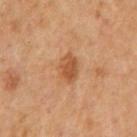| key | value |
|---|---|
| workup | total-body-photography surveillance lesion; no biopsy |
| image-analysis metrics | an average lesion color of about L≈50 a*≈23 b*≈36 (CIELAB), a lesion–skin lightness drop of about 10, and a normalized lesion–skin contrast near 7; a border-irregularity index near 2.5/10 and a peripheral color-asymmetry measure near 1; a detector confidence of about 100 out of 100 that the crop contains a lesion |
| lesion diameter | ≈3.5 mm |
| site | the mid back |
| illumination | cross-polarized |
| patient | male, in their mid- to late 60s |
| image source | ~15 mm crop, total-body skin-cancer survey |